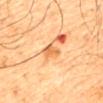The lesion was tiled from a total-body skin photograph and was not biopsied.
A male patient approximately 65 years of age.
From the mid back.
Captured under cross-polarized illumination.
Cropped from a whole-body photographic skin survey; the tile spans about 15 mm.
Measured at roughly 3 mm in maximum diameter.